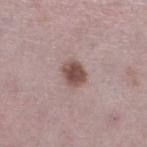Clinical summary:
A male subject about 50 years old. A 15 mm crop from a total-body photograph taken for skin-cancer surveillance. The lesion-visualizer software estimated a footprint of about 6 mm² and a shape eccentricity near 0.5. The analysis additionally found a lesion color around L≈49 a*≈18 b*≈21 in CIELAB, about 14 CIELAB-L* units darker than the surrounding skin, and a normalized border contrast of about 10. The software also gave border irregularity of about 2 on a 0–10 scale, a color-variation rating of about 3/10, and radial color variation of about 1. It also reported an automated nevus-likeness rating near 90 out of 100 and lesion-presence confidence of about 100/100. The tile uses white-light illumination. The lesion is on the left lower leg. About 3 mm across.